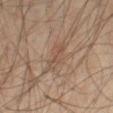notes = imaged on a skin check; not biopsied
subject = male, in their mid- to late 50s
TBP lesion metrics = a border-irregularity rating of about 3.5/10 and a within-lesion color-variation index near 2.5/10
acquisition = ~15 mm crop, total-body skin-cancer survey
lesion diameter = ≈4 mm
body site = the leg
illumination = cross-polarized illumination